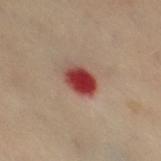Q: Was a biopsy performed?
A: catalogued during a skin exam; not biopsied
Q: How large is the lesion?
A: about 3.5 mm
Q: What is the imaging modality?
A: total-body-photography crop, ~15 mm field of view
Q: What did automated image analysis measure?
A: a footprint of about 7.5 mm², an eccentricity of roughly 0.65, and a shape-asymmetry score of about 0.15 (0 = symmetric); a nevus-likeness score of about 0/100 and a lesion-detection confidence of about 100/100
Q: Lesion location?
A: the left leg
Q: Who is the patient?
A: female, aged approximately 60
Q: Illumination type?
A: cross-polarized illumination